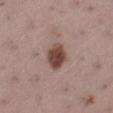Q: Is there a histopathology result?
A: no biopsy performed (imaged during a skin exam)
Q: Where on the body is the lesion?
A: the left lower leg
Q: How was this image acquired?
A: total-body-photography crop, ~15 mm field of view
Q: Patient demographics?
A: female, aged 53–57